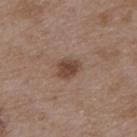  biopsy_status: not biopsied; imaged during a skin examination
  image:
    source: total-body photography crop
    field_of_view_mm: 15
  lesion_size:
    long_diameter_mm_approx: 2.5
  lighting: white-light
  patient:
    sex: male
    age_approx: 50
  site: upper back